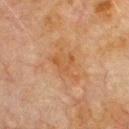| key | value |
|---|---|
| notes | imaged on a skin check; not biopsied |
| subject | male, approximately 80 years of age |
| site | the chest |
| image | total-body-photography crop, ~15 mm field of view |
| tile lighting | cross-polarized illumination |